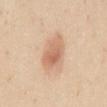Clinical impression: The lesion was tiled from a total-body skin photograph and was not biopsied. Clinical summary: A male subject, roughly 25 years of age. A 15 mm crop from a total-body photograph taken for skin-cancer surveillance. Imaged with white-light lighting. From the mid back. The recorded lesion diameter is about 5 mm.